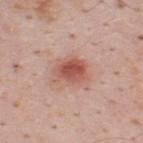The lesion was tiled from a total-body skin photograph and was not biopsied. A lesion tile, about 15 mm wide, cut from a 3D total-body photograph. Captured under white-light illumination. An algorithmic analysis of the crop reported a lesion area of about 8.5 mm² and an outline eccentricity of about 0.6 (0 = round, 1 = elongated). It also reported a mean CIELAB color near L≈54 a*≈26 b*≈27 and roughly 12 lightness units darker than nearby skin. The analysis additionally found border irregularity of about 2 on a 0–10 scale, a within-lesion color-variation index near 5/10, and radial color variation of about 1.5. The software also gave an automated nevus-likeness rating near 95 out of 100 and a detector confidence of about 100 out of 100 that the crop contains a lesion. From the back. The subject is a male in their mid- to late 30s. Longest diameter approximately 3.5 mm.